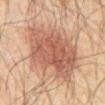{"biopsy_status": "not biopsied; imaged during a skin examination", "site": "abdomen", "lesion_size": {"long_diameter_mm_approx": 8.0}, "patient": {"sex": "male", "age_approx": 60}, "lighting": "cross-polarized", "automated_metrics": {"lesion_detection_confidence_0_100": 100}, "image": {"source": "total-body photography crop", "field_of_view_mm": 15}}